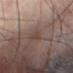A region of skin cropped from a whole-body photographic capture, roughly 15 mm wide.
A male subject, in their mid- to late 60s.
The lesion's longest dimension is about 3 mm.
Imaged with cross-polarized lighting.
Automated tile analysis of the lesion measured an average lesion color of about L≈39 a*≈14 b*≈21 (CIELAB), about 5 CIELAB-L* units darker than the surrounding skin, and a lesion-to-skin contrast of about 5 (normalized; higher = more distinct). The analysis additionally found border irregularity of about 4 on a 0–10 scale, a color-variation rating of about 0/10, and a peripheral color-asymmetry measure near 0. The software also gave a classifier nevus-likeness of about 0/100 and a detector confidence of about 95 out of 100 that the crop contains a lesion.
On the abdomen.